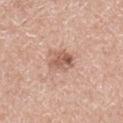Impression: Part of a total-body skin-imaging series; this lesion was reviewed on a skin check and was not flagged for biopsy. Context: The lesion is located on the leg. A male subject, aged 48 to 52. A region of skin cropped from a whole-body photographic capture, roughly 15 mm wide. Imaged with white-light lighting. The lesion-visualizer software estimated an average lesion color of about L≈58 a*≈21 b*≈28 (CIELAB), roughly 11 lightness units darker than nearby skin, and a lesion-to-skin contrast of about 7.5 (normalized; higher = more distinct). And it measured a border-irregularity rating of about 2.5/10 and a color-variation rating of about 5/10. It also reported a lesion-detection confidence of about 100/100.